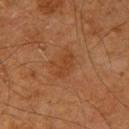| feature | finding |
|---|---|
| workup | no biopsy performed (imaged during a skin exam) |
| illumination | cross-polarized |
| site | the left upper arm |
| imaging modality | total-body-photography crop, ~15 mm field of view |
| patient | male, aged 58–62 |